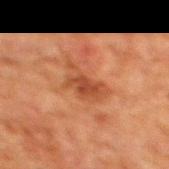| field | value |
|---|---|
| biopsy status | total-body-photography surveillance lesion; no biopsy |
| lighting | cross-polarized |
| acquisition | 15 mm crop, total-body photography |
| size | ~5 mm (longest diameter) |
| subject | male, in their 80s |
| site | the mid back |